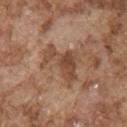notes: catalogued during a skin exam; not biopsied
TBP lesion metrics: roughly 10 lightness units darker than nearby skin and a lesion-to-skin contrast of about 7.5 (normalized; higher = more distinct); border irregularity of about 8 on a 0–10 scale, internal color variation of about 4 on a 0–10 scale, and peripheral color asymmetry of about 1.5; a nevus-likeness score of about 0/100 and a lesion-detection confidence of about 100/100
acquisition: total-body-photography crop, ~15 mm field of view
anatomic site: the chest
illumination: white-light illumination
subject: male, in their mid- to late 70s
lesion diameter: about 5 mm